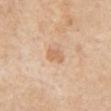  patient:
    sex: male
    age_approx: 85+
  site: upper back
  image:
    source: total-body photography crop
    field_of_view_mm: 15